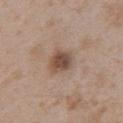Cropped from a whole-body photographic skin survey; the tile spans about 15 mm. A male patient, about 50 years old. The lesion is on the left upper arm.